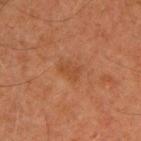The lesion was photographed on a routine skin check and not biopsied; there is no pathology result.